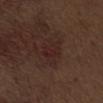- follow-up — catalogued during a skin exam; not biopsied
- anatomic site — the abdomen
- lesion size — about 4 mm
- patient — male, aged around 70
- tile lighting — white-light illumination
- imaging modality — ~15 mm tile from a whole-body skin photo